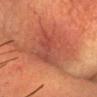The total-body-photography lesion software estimated a lesion area of about 19 mm² and an outline eccentricity of about 0.8 (0 = round, 1 = elongated). And it measured an average lesion color of about L≈42 a*≈27 b*≈28 (CIELAB), roughly 6 lightness units darker than nearby skin, and a normalized border contrast of about 5.5. And it measured a border-irregularity index near 4/10 and peripheral color asymmetry of about 2. The software also gave a classifier nevus-likeness of about 5/100. Longest diameter approximately 6 mm. This image is a 15 mm lesion crop taken from a total-body photograph. A male patient roughly 55 years of age. Imaged with cross-polarized lighting. From the head or neck.